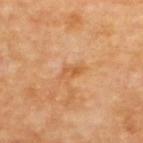{"biopsy_status": "not biopsied; imaged during a skin examination", "patient": {"sex": "male", "age_approx": 70}, "site": "upper back", "lesion_size": {"long_diameter_mm_approx": 2.5}, "image": {"source": "total-body photography crop", "field_of_view_mm": 15}, "lighting": "cross-polarized", "automated_metrics": {"cielab_L": 57, "cielab_a": 24, "cielab_b": 41, "border_irregularity_0_10": 3.5, "color_variation_0_10": 2.5, "peripheral_color_asymmetry": 1.0, "nevus_likeness_0_100": 0}}